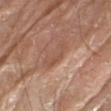Q: What kind of image is this?
A: 15 mm crop, total-body photography
Q: Automated lesion metrics?
A: lesion-presence confidence of about 80/100
Q: Illumination type?
A: white-light
Q: Lesion size?
A: ≈4 mm
Q: Where on the body is the lesion?
A: the right forearm
Q: Patient demographics?
A: male, about 80 years old